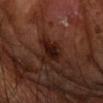The lesion-visualizer software estimated an average lesion color of about L≈17 a*≈20 b*≈20 (CIELAB), a lesion–skin lightness drop of about 10, and a normalized lesion–skin contrast near 12.
A 15 mm close-up extracted from a 3D total-body photography capture.
The patient is a male aged 68 to 72.
Longest diameter approximately 3.5 mm.
The lesion is located on the head or neck.